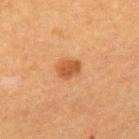lesion size = about 2.5 mm; image = ~15 mm tile from a whole-body skin photo; patient = male, roughly 50 years of age; site = the upper back; illumination = cross-polarized.